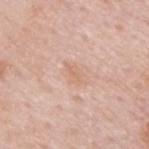{"image": {"source": "total-body photography crop", "field_of_view_mm": 15}, "site": "upper back", "patient": {"sex": "male", "age_approx": 60}}